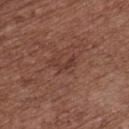A close-up tile cropped from a whole-body skin photograph, about 15 mm across. A male patient, approximately 70 years of age. Measured at roughly 3 mm in maximum diameter. The tile uses white-light illumination. The lesion is located on the chest.